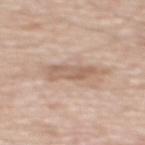<case>
  <lighting>white-light</lighting>
  <image>
    <source>total-body photography crop</source>
    <field_of_view_mm>15</field_of_view_mm>
  </image>
  <lesion_size>
    <long_diameter_mm_approx>5.5</long_diameter_mm_approx>
  </lesion_size>
  <site>back</site>
  <automated_metrics>
    <cielab_L>61</cielab_L>
    <cielab_a>17</cielab_a>
    <cielab_b>28</cielab_b>
    <vs_skin_darker_L>10.0</vs_skin_darker_L>
    <vs_skin_contrast_norm>6.5</vs_skin_contrast_norm>
    <peripheral_color_asymmetry>1.5</peripheral_color_asymmetry>
  </automated_metrics>
  <patient>
    <sex>male</sex>
    <age_approx>70</age_approx>
  </patient>
</case>